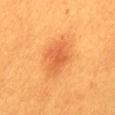Clinical summary: Approximately 3.5 mm at its widest. Imaged with cross-polarized lighting. Located on the mid back. A lesion tile, about 15 mm wide, cut from a 3D total-body photograph. A female subject, aged around 30.